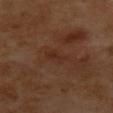Impression: Captured during whole-body skin photography for melanoma surveillance; the lesion was not biopsied. Clinical summary: Approximately 3 mm at its widest. This is a cross-polarized tile. A female subject in their mid- to late 50s. On the upper back. A close-up tile cropped from a whole-body skin photograph, about 15 mm across.